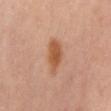Q: Who is the patient?
A: female, in their 50s
Q: What lighting was used for the tile?
A: cross-polarized
Q: Where on the body is the lesion?
A: the mid back
Q: What is the lesion's diameter?
A: ≈5 mm
Q: What is the imaging modality?
A: ~15 mm crop, total-body skin-cancer survey